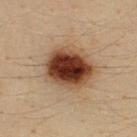Part of a total-body skin-imaging series; this lesion was reviewed on a skin check and was not flagged for biopsy.
The tile uses cross-polarized illumination.
A 15 mm crop from a total-body photograph taken for skin-cancer surveillance.
The subject is a male roughly 35 years of age.
Located on the upper back.
The total-body-photography lesion software estimated an area of roughly 20 mm², an outline eccentricity of about 0.6 (0 = round, 1 = elongated), and a symmetry-axis asymmetry near 0.1.
Measured at roughly 5.5 mm in maximum diameter.